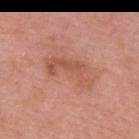The lesion was photographed on a routine skin check and not biopsied; there is no pathology result.
A male subject aged 73 to 77.
The recorded lesion diameter is about 6.5 mm.
A 15 mm close-up extracted from a 3D total-body photography capture.
Located on the back.
This is a white-light tile.